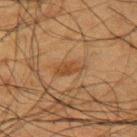No biopsy was performed on this lesion — it was imaged during a full skin examination and was not determined to be concerning. From the upper back. The recorded lesion diameter is about 3.5 mm. A male subject, aged around 60. Automated tile analysis of the lesion measured a footprint of about 4 mm² and an eccentricity of roughly 0.85. It also reported an average lesion color of about L≈36 a*≈17 b*≈30 (CIELAB), roughly 6 lightness units darker than nearby skin, and a lesion-to-skin contrast of about 6.5 (normalized; higher = more distinct). The software also gave border irregularity of about 3.5 on a 0–10 scale, a color-variation rating of about 2/10, and a peripheral color-asymmetry measure near 0.5. A roughly 15 mm field-of-view crop from a total-body skin photograph. The tile uses cross-polarized illumination.